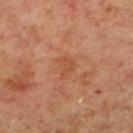{"biopsy_status": "not biopsied; imaged during a skin examination", "patient": {"sex": "male", "age_approx": 60}, "site": "left lower leg", "image": {"source": "total-body photography crop", "field_of_view_mm": 15}}